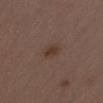biopsy status — total-body-photography surveillance lesion; no biopsy
anatomic site — the chest
subject — male, in their 40s
image source — total-body-photography crop, ~15 mm field of view
lesion diameter — ~2.5 mm (longest diameter)
tile lighting — white-light